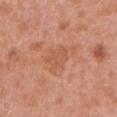workup = no biopsy performed (imaged during a skin exam) | patient = female, aged around 40 | imaging modality = ~15 mm tile from a whole-body skin photo | anatomic site = the left upper arm | image-analysis metrics = a shape eccentricity near 0.7 and a symmetry-axis asymmetry near 0.45; border irregularity of about 5.5 on a 0–10 scale, internal color variation of about 2 on a 0–10 scale, and a peripheral color-asymmetry measure near 1.